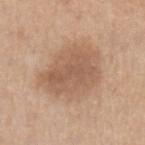notes: total-body-photography surveillance lesion; no biopsy
diameter: ≈7.5 mm
location: the left upper arm
TBP lesion metrics: a lesion area of about 27 mm², a shape eccentricity near 0.7, and a shape-asymmetry score of about 0.2 (0 = symmetric); a lesion color around L≈57 a*≈19 b*≈31 in CIELAB, about 10 CIELAB-L* units darker than the surrounding skin, and a normalized lesion–skin contrast near 6.5; a border-irregularity index near 2.5/10, internal color variation of about 3.5 on a 0–10 scale, and a peripheral color-asymmetry measure near 1; a classifier nevus-likeness of about 15/100 and lesion-presence confidence of about 100/100
patient: male, aged 58 to 62
acquisition: 15 mm crop, total-body photography